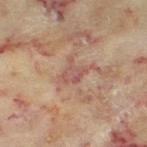Assessment:
Part of a total-body skin-imaging series; this lesion was reviewed on a skin check and was not flagged for biopsy.
Context:
The patient is a female aged 58 to 62. About 3 mm across. From the leg. A 15 mm close-up extracted from a 3D total-body photography capture. The total-body-photography lesion software estimated a border-irregularity rating of about 5.5/10 and a color-variation rating of about 3/10. The analysis additionally found a nevus-likeness score of about 0/100 and lesion-presence confidence of about 70/100. The tile uses cross-polarized illumination.